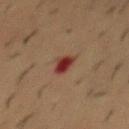Recorded during total-body skin imaging; not selected for excision or biopsy.
The tile uses cross-polarized illumination.
The lesion is on the mid back.
A male patient aged approximately 55.
A 15 mm crop from a total-body photograph taken for skin-cancer surveillance.
The lesion's longest dimension is about 3 mm.